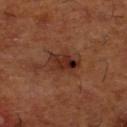Impression: The lesion was tiled from a total-body skin photograph and was not biopsied.